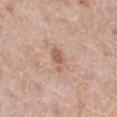Assessment:
Captured during whole-body skin photography for melanoma surveillance; the lesion was not biopsied.
Acquisition and patient details:
Cropped from a total-body skin-imaging series; the visible field is about 15 mm. This is a white-light tile. On the right lower leg. An algorithmic analysis of the crop reported an average lesion color of about L≈58 a*≈21 b*≈30 (CIELAB) and a lesion–skin lightness drop of about 10. The software also gave a color-variation rating of about 2.5/10 and a peripheral color-asymmetry measure near 1. A female subject about 65 years old.